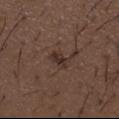A close-up tile cropped from a whole-body skin photograph, about 15 mm across. A male subject aged 48 to 52. On the mid back.